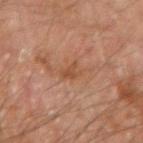Captured during whole-body skin photography for melanoma surveillance; the lesion was not biopsied. This image is a 15 mm lesion crop taken from a total-body photograph. A male subject, aged 43 to 47. About 2.5 mm across. The lesion is located on the left forearm.